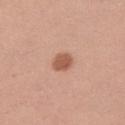biopsy status: catalogued during a skin exam; not biopsied | TBP lesion metrics: a footprint of about 4.5 mm² and an outline eccentricity of about 0.55 (0 = round, 1 = elongated); a classifier nevus-likeness of about 100/100 and lesion-presence confidence of about 100/100 | patient: male, in their 30s | tile lighting: white-light illumination | image source: total-body-photography crop, ~15 mm field of view | anatomic site: the left upper arm | diameter: ~2.5 mm (longest diameter).